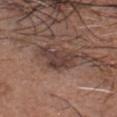No biopsy was performed on this lesion — it was imaged during a full skin examination and was not determined to be concerning. The tile uses white-light illumination. Cropped from a whole-body photographic skin survey; the tile spans about 15 mm. A male patient, aged 53–57. The total-body-photography lesion software estimated a lesion color around L≈39 a*≈17 b*≈21 in CIELAB and roughly 9 lightness units darker than nearby skin. And it measured a border-irregularity index near 5/10, internal color variation of about 3 on a 0–10 scale, and peripheral color asymmetry of about 1. On the head or neck.